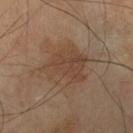  biopsy_status: not biopsied; imaged during a skin examination
  image:
    source: total-body photography crop
    field_of_view_mm: 15
  lighting: cross-polarized
  lesion_size:
    long_diameter_mm_approx: 5.5
  automated_metrics:
    area_mm2_approx: 19.0
    eccentricity: 0.5
  patient:
    sex: male
    age_approx: 65
  site: right lower leg